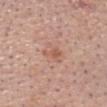This lesion was catalogued during total-body skin photography and was not selected for biopsy. Cropped from a total-body skin-imaging series; the visible field is about 15 mm. The tile uses white-light illumination. The patient is a male in their 60s. Automated tile analysis of the lesion measured an outline eccentricity of about 0.8 (0 = round, 1 = elongated) and a symmetry-axis asymmetry near 0.5. The analysis additionally found about 8 CIELAB-L* units darker than the surrounding skin and a normalized border contrast of about 5.5. It also reported a border-irregularity rating of about 5/10, a color-variation rating of about 1.5/10, and a peripheral color-asymmetry measure near 0.5. Measured at roughly 2.5 mm in maximum diameter. Located on the head or neck.